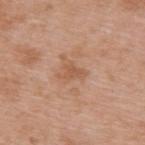{
  "biopsy_status": "not biopsied; imaged during a skin examination",
  "image": {
    "source": "total-body photography crop",
    "field_of_view_mm": 15
  },
  "site": "back",
  "patient": {
    "sex": "female",
    "age_approx": 40
  }
}